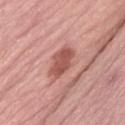workup: no biopsy performed (imaged during a skin exam); illumination: white-light illumination; location: the leg; patient: female, in their mid-40s; image source: ~15 mm tile from a whole-body skin photo.